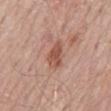Recorded during total-body skin imaging; not selected for excision or biopsy.
A lesion tile, about 15 mm wide, cut from a 3D total-body photograph.
The recorded lesion diameter is about 3 mm.
The lesion is located on the mid back.
This is a white-light tile.
The lesion-visualizer software estimated a lesion area of about 5.5 mm², a shape eccentricity near 0.75, and two-axis asymmetry of about 0.3. The analysis additionally found internal color variation of about 2.5 on a 0–10 scale and a peripheral color-asymmetry measure near 1.
The patient is a male aged around 70.